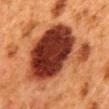Assessment:
The lesion was photographed on a routine skin check and not biopsied; there is no pathology result.
Acquisition and patient details:
The patient is a female roughly 50 years of age. A 15 mm close-up tile from a total-body photography series done for melanoma screening. From the mid back.